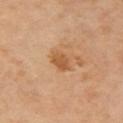workup: no biopsy performed (imaged during a skin exam) | lesion diameter: about 3 mm | location: the left arm | imaging modality: ~15 mm crop, total-body skin-cancer survey | image-analysis metrics: a shape-asymmetry score of about 0.25 (0 = symmetric); a mean CIELAB color near L≈53 a*≈22 b*≈37, a lesion–skin lightness drop of about 9, and a normalized border contrast of about 7; border irregularity of about 2.5 on a 0–10 scale, internal color variation of about 2 on a 0–10 scale, and a peripheral color-asymmetry measure near 0.5; lesion-presence confidence of about 100/100 | patient: female, roughly 65 years of age | lighting: cross-polarized illumination.